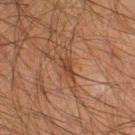Case summary:
- lighting — cross-polarized
- subject — male, in their mid-30s
- location — the right thigh
- image source — 15 mm crop, total-body photography